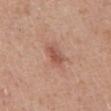This lesion was catalogued during total-body skin photography and was not selected for biopsy. A male subject, in their 50s. The recorded lesion diameter is about 2.5 mm. Imaged with white-light lighting. From the front of the torso. Cropped from a total-body skin-imaging series; the visible field is about 15 mm.